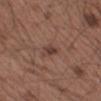This is a white-light tile. The patient is a male about 55 years old. Cropped from a total-body skin-imaging series; the visible field is about 15 mm. Approximately 3 mm at its widest. The lesion is on the arm.